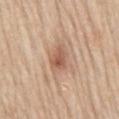Q: Was a biopsy performed?
A: no biopsy performed (imaged during a skin exam)
Q: How large is the lesion?
A: ≈3 mm
Q: What is the imaging modality?
A: ~15 mm crop, total-body skin-cancer survey
Q: Patient demographics?
A: male, roughly 65 years of age
Q: How was the tile lit?
A: white-light illumination
Q: Automated lesion metrics?
A: an area of roughly 5 mm², an outline eccentricity of about 0.7 (0 = round, 1 = elongated), and a shape-asymmetry score of about 0.3 (0 = symmetric); border irregularity of about 3 on a 0–10 scale and peripheral color asymmetry of about 1.5; an automated nevus-likeness rating near 80 out of 100
Q: Lesion location?
A: the mid back